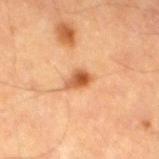follow-up — catalogued during a skin exam; not biopsied | subject — male, aged 83 or older | body site — the left thigh | tile lighting — cross-polarized | image source — 15 mm crop, total-body photography.